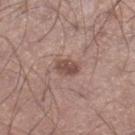Captured during whole-body skin photography for melanoma surveillance; the lesion was not biopsied. An algorithmic analysis of the crop reported a lesion area of about 4 mm², an eccentricity of roughly 0.7, and a shape-asymmetry score of about 0.2 (0 = symmetric). And it measured a border-irregularity index near 2/10, internal color variation of about 2 on a 0–10 scale, and radial color variation of about 0.5. It also reported an automated nevus-likeness rating near 60 out of 100 and a detector confidence of about 100 out of 100 that the crop contains a lesion. A lesion tile, about 15 mm wide, cut from a 3D total-body photograph. The subject is a male approximately 40 years of age. The tile uses white-light illumination. The lesion is located on the right lower leg.